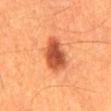Captured during whole-body skin photography for melanoma surveillance; the lesion was not biopsied. The lesion's longest dimension is about 5 mm. A roughly 15 mm field-of-view crop from a total-body skin photograph. A male subject, aged 58–62. The lesion is on the mid back. The tile uses cross-polarized illumination.